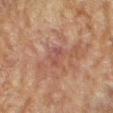– follow-up · total-body-photography surveillance lesion; no biopsy
– patient · female, approximately 80 years of age
– automated lesion analysis · a lesion area of about 2.5 mm², a shape eccentricity near 0.95, and two-axis asymmetry of about 0.3; a lesion color around L≈42 a*≈21 b*≈23 in CIELAB and a normalized lesion–skin contrast near 5
– tile lighting · cross-polarized illumination
– body site · the right forearm
– lesion diameter · about 3 mm
– image · ~15 mm crop, total-body skin-cancer survey